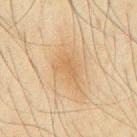Recorded during total-body skin imaging; not selected for excision or biopsy. The subject is a male roughly 65 years of age. An algorithmic analysis of the crop reported a footprint of about 8 mm², an outline eccentricity of about 0.8 (0 = round, 1 = elongated), and a symmetry-axis asymmetry near 0.35. And it measured a border-irregularity index near 4/10, a within-lesion color-variation index near 2.5/10, and radial color variation of about 1. The analysis additionally found a nevus-likeness score of about 15/100 and lesion-presence confidence of about 100/100. A 15 mm close-up tile from a total-body photography series done for melanoma screening. The lesion is on the chest.